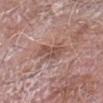– workup · catalogued during a skin exam; not biopsied
– location · the right forearm
– lesion diameter · ≈4 mm
– subject · male, about 75 years old
– illumination · white-light
– automated metrics · a footprint of about 6.5 mm² and a symmetry-axis asymmetry near 0.3
– image source · 15 mm crop, total-body photography